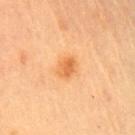Assessment: The lesion was photographed on a routine skin check and not biopsied; there is no pathology result. Context: A roughly 15 mm field-of-view crop from a total-body skin photograph. Automated tile analysis of the lesion measured a footprint of about 4 mm² and two-axis asymmetry of about 0.2. The analysis additionally found a color-variation rating of about 2.5/10 and a peripheral color-asymmetry measure near 1. The software also gave a classifier nevus-likeness of about 90/100 and a lesion-detection confidence of about 100/100. On the chest. A female patient approximately 60 years of age.